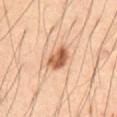The lesion was photographed on a routine skin check and not biopsied; there is no pathology result.
The total-body-photography lesion software estimated an outline eccentricity of about 0.75 (0 = round, 1 = elongated) and a shape-asymmetry score of about 0.2 (0 = symmetric). The software also gave a mean CIELAB color near L≈58 a*≈23 b*≈35, a lesion–skin lightness drop of about 15, and a lesion-to-skin contrast of about 10 (normalized; higher = more distinct). The software also gave peripheral color asymmetry of about 2.5.
Cropped from a whole-body photographic skin survey; the tile spans about 15 mm.
The recorded lesion diameter is about 3.5 mm.
Imaged with cross-polarized lighting.
Located on the abdomen.
A male subject approximately 60 years of age.